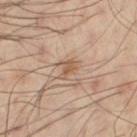Impression: The lesion was tiled from a total-body skin photograph and was not biopsied. Acquisition and patient details: Measured at roughly 2.5 mm in maximum diameter. The lesion is on the abdomen. A close-up tile cropped from a whole-body skin photograph, about 15 mm across. The tile uses cross-polarized illumination. The patient is a male in their 50s.